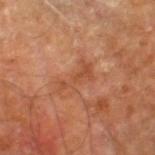workup=no biopsy performed (imaged during a skin exam)